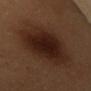Imaged during a routine full-body skin examination; the lesion was not biopsied and no histopathology is available. The lesion is located on the mid back. A 15 mm crop from a total-body photograph taken for skin-cancer surveillance. Automated image analysis of the tile measured a lesion area of about 30 mm² and two-axis asymmetry of about 0.2. The software also gave an average lesion color of about L≈22 a*≈18 b*≈23 (CIELAB), a lesion–skin lightness drop of about 11, and a normalized border contrast of about 12. And it measured a within-lesion color-variation index near 4/10. A female patient aged 48 to 52. This is a cross-polarized tile.